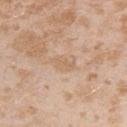The lesion was photographed on a routine skin check and not biopsied; there is no pathology result. Approximately 3 mm at its widest. A 15 mm close-up extracted from a 3D total-body photography capture. The tile uses white-light illumination. Automated tile analysis of the lesion measured a lesion color around L≈63 a*≈17 b*≈33 in CIELAB, about 6 CIELAB-L* units darker than the surrounding skin, and a normalized lesion–skin contrast near 4.5. And it measured an automated nevus-likeness rating near 0 out of 100 and lesion-presence confidence of about 100/100. A female subject, in their mid-20s. The lesion is located on the left upper arm.